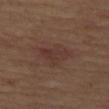| feature | finding |
|---|---|
| follow-up | imaged on a skin check; not biopsied |
| site | the left thigh |
| subject | female, in their 40s |
| acquisition | total-body-photography crop, ~15 mm field of view |